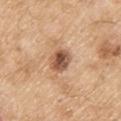Q: Is there a histopathology result?
A: total-body-photography surveillance lesion; no biopsy
Q: Lesion size?
A: about 3 mm
Q: What did automated image analysis measure?
A: a mean CIELAB color near L≈54 a*≈21 b*≈32, roughly 15 lightness units darker than nearby skin, and a normalized border contrast of about 10; an automated nevus-likeness rating near 70 out of 100 and a detector confidence of about 100 out of 100 that the crop contains a lesion
Q: Patient demographics?
A: male, in their 70s
Q: Where on the body is the lesion?
A: the upper back
Q: How was this image acquired?
A: ~15 mm tile from a whole-body skin photo